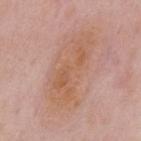Q: Is there a histopathology result?
A: no biopsy performed (imaged during a skin exam)
Q: Automated lesion metrics?
A: a classifier nevus-likeness of about 0/100
Q: How was the tile lit?
A: white-light
Q: What are the patient's age and sex?
A: male, roughly 55 years of age
Q: What kind of image is this?
A: 15 mm crop, total-body photography
Q: Lesion location?
A: the mid back
Q: Lesion size?
A: ≈9.5 mm